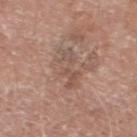notes — no biopsy performed (imaged during a skin exam)
TBP lesion metrics — an outline eccentricity of about 0.9 (0 = round, 1 = elongated) and a shape-asymmetry score of about 0.3 (0 = symmetric); roughly 7 lightness units darker than nearby skin and a lesion-to-skin contrast of about 5.5 (normalized; higher = more distinct); a border-irregularity rating of about 6/10, internal color variation of about 5.5 on a 0–10 scale, and a peripheral color-asymmetry measure near 2; a lesion-detection confidence of about 85/100
location — the left lower leg
subject — male, aged around 80
acquisition — ~15 mm crop, total-body skin-cancer survey
illumination — white-light illumination